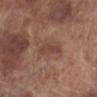This lesion was catalogued during total-body skin photography and was not selected for biopsy. Longest diameter approximately 6 mm. This is a white-light tile. The subject is a male in their 70s. Located on the left lower leg. A region of skin cropped from a whole-body photographic capture, roughly 15 mm wide.